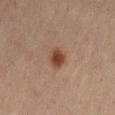Recorded during total-body skin imaging; not selected for excision or biopsy. A close-up tile cropped from a whole-body skin photograph, about 15 mm across. This is a cross-polarized tile. The subject is a female aged 58–62. On the abdomen. The lesion's longest dimension is about 3 mm.